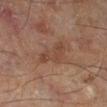Case summary:
- image source — ~15 mm tile from a whole-body skin photo
- illumination — cross-polarized illumination
- patient — male, aged approximately 65
- lesion size — about 4.5 mm
- anatomic site — the left lower leg